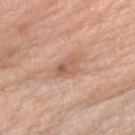Notes:
* follow-up · total-body-photography surveillance lesion; no biopsy
* patient · female, approximately 70 years of age
* image source · total-body-photography crop, ~15 mm field of view
* site · the right forearm
* diameter · about 4 mm